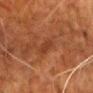Clinical impression:
This lesion was catalogued during total-body skin photography and was not selected for biopsy.
Acquisition and patient details:
The lesion is located on the front of the torso. The recorded lesion diameter is about 2.5 mm. A male patient, aged 78–82. A 15 mm close-up tile from a total-body photography series done for melanoma screening. Imaged with cross-polarized lighting. Automated tile analysis of the lesion measured an area of roughly 2 mm² and a symmetry-axis asymmetry near 0.4.